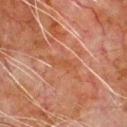No biopsy was performed on this lesion — it was imaged during a full skin examination and was not determined to be concerning.
A male patient aged approximately 80.
On the chest.
A close-up tile cropped from a whole-body skin photograph, about 15 mm across.
Captured under cross-polarized illumination.
The total-body-photography lesion software estimated a border-irregularity rating of about 5/10, a color-variation rating of about 0/10, and a peripheral color-asymmetry measure near 0.
The recorded lesion diameter is about 3.5 mm.